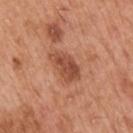Findings:
• workup: catalogued during a skin exam; not biopsied
• patient: male, in their mid- to late 50s
• acquisition: 15 mm crop, total-body photography
• lesion size: about 4.5 mm
• body site: the right upper arm
• illumination: white-light
• image-analysis metrics: a footprint of about 8.5 mm²; a normalized lesion–skin contrast near 8